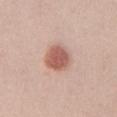Case summary:
- location · the front of the torso
- lighting · white-light illumination
- automated metrics · a mean CIELAB color near L≈58 a*≈24 b*≈27, about 13 CIELAB-L* units darker than the surrounding skin, and a lesion-to-skin contrast of about 9 (normalized; higher = more distinct)
- diameter · about 3.5 mm
- imaging modality · ~15 mm tile from a whole-body skin photo
- patient · male, aged 23 to 27